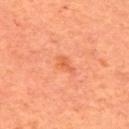Imaged during a routine full-body skin examination; the lesion was not biopsied and no histopathology is available. A roughly 15 mm field-of-view crop from a total-body skin photograph. The tile uses cross-polarized illumination. Located on the upper back. Approximately 3 mm at its widest. The total-body-photography lesion software estimated a mean CIELAB color near L≈56 a*≈30 b*≈39, roughly 7 lightness units darker than nearby skin, and a lesion-to-skin contrast of about 5.5 (normalized; higher = more distinct). And it measured internal color variation of about 1.5 on a 0–10 scale and radial color variation of about 0.5. The software also gave a detector confidence of about 100 out of 100 that the crop contains a lesion. The subject is a male aged 63 to 67.